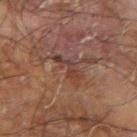Clinical impression:
Part of a total-body skin-imaging series; this lesion was reviewed on a skin check and was not flagged for biopsy.
Acquisition and patient details:
The patient is a male aged 68 to 72. Cropped from a total-body skin-imaging series; the visible field is about 15 mm. Automated tile analysis of the lesion measured a lesion area of about 5 mm² and two-axis asymmetry of about 0.35. The software also gave roughly 6 lightness units darker than nearby skin and a normalized lesion–skin contrast near 6.5. And it measured a border-irregularity rating of about 6/10. The software also gave a classifier nevus-likeness of about 0/100 and lesion-presence confidence of about 80/100. This is a cross-polarized tile. Located on the left forearm.